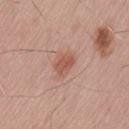Impression:
The lesion was tiled from a total-body skin photograph and was not biopsied.
Context:
A roughly 15 mm field-of-view crop from a total-body skin photograph. The subject is a male about 55 years old. The lesion is located on the lower back.